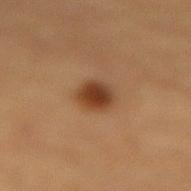Part of a total-body skin-imaging series; this lesion was reviewed on a skin check and was not flagged for biopsy. Located on the lower back. Captured under cross-polarized illumination. The subject is a male in their mid-80s or older. The lesion's longest dimension is about 3 mm. A close-up tile cropped from a whole-body skin photograph, about 15 mm across.